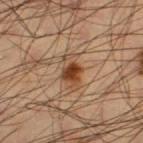Findings:
- workup: catalogued during a skin exam; not biopsied
- lesion diameter: ≈4 mm
- image: ~15 mm tile from a whole-body skin photo
- patient: male, in their 60s
- body site: the left thigh
- lighting: cross-polarized